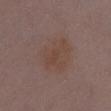* notes — catalogued during a skin exam; not biopsied
* lighting — white-light
* automated lesion analysis — a mean CIELAB color near L≈44 a*≈16 b*≈23, roughly 4 lightness units darker than nearby skin, and a normalized lesion–skin contrast near 5; border irregularity of about 2 on a 0–10 scale, a color-variation rating of about 3/10, and radial color variation of about 1; a classifier nevus-likeness of about 0/100 and a lesion-detection confidence of about 100/100
* diameter — ≈5 mm
* patient — female, approximately 30 years of age
* location — the front of the torso
* image — 15 mm crop, total-body photography